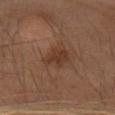Findings:
• body site · the head or neck
• image source · 15 mm crop, total-body photography
• automated metrics · a lesion area of about 6.5 mm² and a shape-asymmetry score of about 0.3 (0 = symmetric); an average lesion color of about L≈29 a*≈16 b*≈24 (CIELAB), about 6 CIELAB-L* units darker than the surrounding skin, and a normalized lesion–skin contrast near 6.5; a border-irregularity rating of about 3/10, a within-lesion color-variation index near 2/10, and a peripheral color-asymmetry measure near 0.5
• tile lighting · cross-polarized
• subject · female, roughly 55 years of age